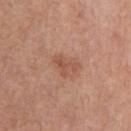A region of skin cropped from a whole-body photographic capture, roughly 15 mm wide. Imaged with white-light lighting. A male patient, about 75 years old. On the chest. The lesion's longest dimension is about 3 mm. An algorithmic analysis of the crop reported an area of roughly 5 mm², an outline eccentricity of about 0.6 (0 = round, 1 = elongated), and a symmetry-axis asymmetry near 0.45. And it measured roughly 7 lightness units darker than nearby skin and a normalized lesion–skin contrast near 5.5. It also reported an automated nevus-likeness rating near 20 out of 100 and a detector confidence of about 100 out of 100 that the crop contains a lesion.